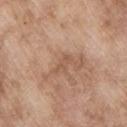Located on the upper back. A 15 mm close-up extracted from a 3D total-body photography capture. A male patient aged around 65.